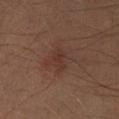notes — no biopsy performed (imaged during a skin exam); diameter — about 3 mm; anatomic site — the left lower leg; subject — male, aged 38–42; imaging modality — total-body-photography crop, ~15 mm field of view; illumination — cross-polarized.